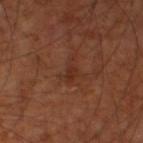A 15 mm close-up extracted from a 3D total-body photography capture. About 3.5 mm across. The tile uses cross-polarized illumination. Located on the left thigh. A male patient roughly 60 years of age.